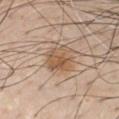Clinical impression: This lesion was catalogued during total-body skin photography and was not selected for biopsy. Acquisition and patient details: The lesion is on the chest. The lesion-visualizer software estimated a lesion area of about 9 mm², an outline eccentricity of about 0.45 (0 = round, 1 = elongated), and a symmetry-axis asymmetry near 0.2. The software also gave a normalized border contrast of about 7. It also reported a border-irregularity rating of about 2.5/10 and a color-variation rating of about 5/10. It also reported a nevus-likeness score of about 90/100 and a lesion-detection confidence of about 100/100. Captured under white-light illumination. A male subject, in their 60s. Cropped from a whole-body photographic skin survey; the tile spans about 15 mm. Approximately 3.5 mm at its widest.